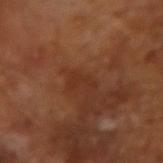workup=imaged on a skin check; not biopsied
image-analysis metrics=roughly 5 lightness units darker than nearby skin and a normalized lesion–skin contrast near 5; a border-irregularity index near 7.5/10 and a peripheral color-asymmetry measure near 0
diameter=≈2.5 mm
patient=male, approximately 65 years of age
illumination=cross-polarized illumination
acquisition=~15 mm crop, total-body skin-cancer survey